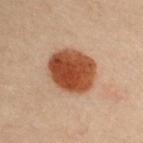| feature | finding |
|---|---|
| notes | total-body-photography surveillance lesion; no biopsy |
| imaging modality | 15 mm crop, total-body photography |
| TBP lesion metrics | a lesion area of about 20 mm², a shape eccentricity near 0.55, and two-axis asymmetry of about 0.1; a lesion color around L≈38 a*≈22 b*≈29 in CIELAB and about 15 CIELAB-L* units darker than the surrounding skin; a classifier nevus-likeness of about 100/100 |
| lesion size | ≈5 mm |
| lighting | cross-polarized illumination |
| anatomic site | the chest |
| subject | female, aged 28 to 32 |